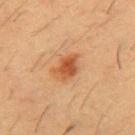Impression:
The lesion was photographed on a routine skin check and not biopsied; there is no pathology result.
Clinical summary:
This image is a 15 mm lesion crop taken from a total-body photograph. Longest diameter approximately 3.5 mm. A male patient aged 53–57. The lesion is on the chest. Captured under cross-polarized illumination. An algorithmic analysis of the crop reported a footprint of about 7 mm² and a symmetry-axis asymmetry near 0.2. And it measured border irregularity of about 2.5 on a 0–10 scale and a peripheral color-asymmetry measure near 1.5. It also reported a nevus-likeness score of about 95/100 and lesion-presence confidence of about 100/100.